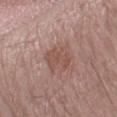| key | value |
|---|---|
| workup | no biopsy performed (imaged during a skin exam) |
| patient | male, in their mid-50s |
| illumination | white-light illumination |
| site | the left forearm |
| image source | ~15 mm tile from a whole-body skin photo |